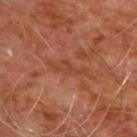Assessment:
Imaged during a routine full-body skin examination; the lesion was not biopsied and no histopathology is available.
Clinical summary:
The lesion's longest dimension is about 4.5 mm. This is a cross-polarized tile. Located on the chest. The lesion-visualizer software estimated a footprint of about 5 mm² and a shape-asymmetry score of about 0.35 (0 = symmetric). It also reported a mean CIELAB color near L≈42 a*≈25 b*≈32 and a lesion-to-skin contrast of about 5.5 (normalized; higher = more distinct). The software also gave a border-irregularity rating of about 4.5/10 and internal color variation of about 1.5 on a 0–10 scale. And it measured a nevus-likeness score of about 0/100 and a detector confidence of about 50 out of 100 that the crop contains a lesion. The subject is a male aged 58 to 62. Cropped from a whole-body photographic skin survey; the tile spans about 15 mm.